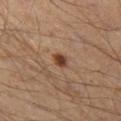{
  "image": {
    "source": "total-body photography crop",
    "field_of_view_mm": 15
  },
  "lesion_size": {
    "long_diameter_mm_approx": 2.0
  },
  "site": "left thigh",
  "lighting": "cross-polarized",
  "patient": {
    "sex": "male",
    "age_approx": 45
  }
}